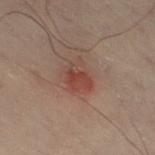Q: Was this lesion biopsied?
A: no biopsy performed (imaged during a skin exam)
Q: What lighting was used for the tile?
A: cross-polarized
Q: How was this image acquired?
A: 15 mm crop, total-body photography
Q: What did automated image analysis measure?
A: an outline eccentricity of about 0.7 (0 = round, 1 = elongated); a color-variation rating of about 6.5/10; a nevus-likeness score of about 40/100 and a detector confidence of about 100 out of 100 that the crop contains a lesion
Q: Who is the patient?
A: male, aged 48–52
Q: What is the lesion's diameter?
A: ~4 mm (longest diameter)
Q: What is the anatomic site?
A: the left leg